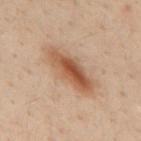Clinical impression:
No biopsy was performed on this lesion — it was imaged during a full skin examination and was not determined to be concerning.
Acquisition and patient details:
The lesion is located on the back. The tile uses cross-polarized illumination. A 15 mm close-up extracted from a 3D total-body photography capture. Automated tile analysis of the lesion measured about 10 CIELAB-L* units darker than the surrounding skin. The analysis additionally found lesion-presence confidence of about 100/100. A male subject, approximately 30 years of age. Longest diameter approximately 6.5 mm.